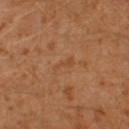No biopsy was performed on this lesion — it was imaged during a full skin examination and was not determined to be concerning. The lesion is located on the left lower leg. Automated image analysis of the tile measured a lesion color around L≈45 a*≈22 b*≈35 in CIELAB, a lesion–skin lightness drop of about 5, and a normalized lesion–skin contrast near 4.5. The analysis additionally found a border-irregularity rating of about 6/10, a within-lesion color-variation index near 0/10, and radial color variation of about 0. It also reported a nevus-likeness score of about 0/100. A male subject, aged around 30. A region of skin cropped from a whole-body photographic capture, roughly 15 mm wide. This is a cross-polarized tile. The recorded lesion diameter is about 3 mm.